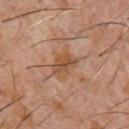– follow-up — imaged on a skin check; not biopsied
– size — about 4 mm
– image — ~15 mm tile from a whole-body skin photo
– location — the chest
– subject — male, aged around 60
– lighting — cross-polarized
– TBP lesion metrics — an area of roughly 8.5 mm², an outline eccentricity of about 0.65 (0 = round, 1 = elongated), and a shape-asymmetry score of about 0.25 (0 = symmetric); roughly 7 lightness units darker than nearby skin and a lesion-to-skin contrast of about 6.5 (normalized; higher = more distinct); a border-irregularity rating of about 3/10, a within-lesion color-variation index near 5.5/10, and radial color variation of about 2